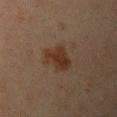Captured during whole-body skin photography for melanoma surveillance; the lesion was not biopsied. Imaged with cross-polarized lighting. The patient is a female in their mid- to late 40s. Automated image analysis of the tile measured a shape eccentricity near 0.75 and a shape-asymmetry score of about 0.35 (0 = symmetric). The analysis additionally found an automated nevus-likeness rating near 75 out of 100 and a lesion-detection confidence of about 100/100. Approximately 4 mm at its widest. A lesion tile, about 15 mm wide, cut from a 3D total-body photograph. Located on the left upper arm.